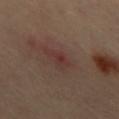follow-up: total-body-photography surveillance lesion; no biopsy | lesion diameter: ≈3.5 mm | anatomic site: the chest | lighting: cross-polarized illumination | image source: total-body-photography crop, ~15 mm field of view.